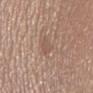notes: imaged on a skin check; not biopsied | body site: the left lower leg | subject: female, roughly 40 years of age | imaging modality: ~15 mm crop, total-body skin-cancer survey | diameter: ~3 mm (longest diameter) | illumination: white-light | automated metrics: an outline eccentricity of about 0.85 (0 = round, 1 = elongated) and a shape-asymmetry score of about 0.45 (0 = symmetric); a classifier nevus-likeness of about 0/100 and a detector confidence of about 95 out of 100 that the crop contains a lesion.